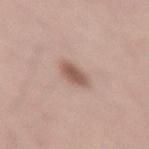Cropped from a whole-body photographic skin survey; the tile spans about 15 mm.
Automated tile analysis of the lesion measured an eccentricity of roughly 0.65 and two-axis asymmetry of about 0.2. The software also gave a lesion color around L≈56 a*≈19 b*≈25 in CIELAB, about 12 CIELAB-L* units darker than the surrounding skin, and a lesion-to-skin contrast of about 8 (normalized; higher = more distinct). The software also gave a border-irregularity rating of about 2/10, a color-variation rating of about 2/10, and a peripheral color-asymmetry measure near 0.5. And it measured an automated nevus-likeness rating near 95 out of 100.
Located on the lower back.
The lesion's longest dimension is about 3 mm.
Captured under white-light illumination.
The subject is a male aged around 50.